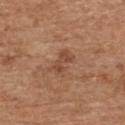biopsy_status: not biopsied; imaged during a skin examination
site: upper back
automated_metrics:
  area_mm2_approx: 4.0
  eccentricity: 0.85
  shape_asymmetry: 0.35
  nevus_likeness_0_100: 5
patient:
  sex: male
  age_approx: 70
lesion_size:
  long_diameter_mm_approx: 3.0
lighting: white-light
image:
  source: total-body photography crop
  field_of_view_mm: 15